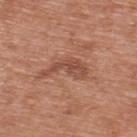This lesion was catalogued during total-body skin photography and was not selected for biopsy. A region of skin cropped from a whole-body photographic capture, roughly 15 mm wide. From the back. Captured under white-light illumination. Automated tile analysis of the lesion measured an area of roughly 6.5 mm², an eccentricity of roughly 0.9, and a shape-asymmetry score of about 0.5 (0 = symmetric). The analysis additionally found an average lesion color of about L≈49 a*≈24 b*≈29 (CIELAB) and about 9 CIELAB-L* units darker than the surrounding skin. About 5 mm across. A male patient, roughly 70 years of age.